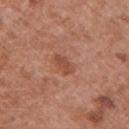{"biopsy_status": "not biopsied; imaged during a skin examination", "lesion_size": {"long_diameter_mm_approx": 3.0}, "lighting": "white-light", "image": {"source": "total-body photography crop", "field_of_view_mm": 15}, "site": "left upper arm", "patient": {"sex": "female", "age_approx": 40}}